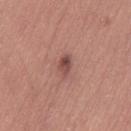This lesion was catalogued during total-body skin photography and was not selected for biopsy. The lesion-visualizer software estimated an eccentricity of roughly 0.85 and a symmetry-axis asymmetry near 0.3. The software also gave a mean CIELAB color near L≈48 a*≈23 b*≈23, roughly 11 lightness units darker than nearby skin, and a lesion-to-skin contrast of about 8 (normalized; higher = more distinct). It also reported border irregularity of about 3 on a 0–10 scale, a color-variation rating of about 5/10, and peripheral color asymmetry of about 2.5. The analysis additionally found a classifier nevus-likeness of about 80/100 and a lesion-detection confidence of about 100/100. Measured at roughly 3 mm in maximum diameter. This image is a 15 mm lesion crop taken from a total-body photograph. A female patient, aged around 25. Located on the right thigh. Imaged with white-light lighting.